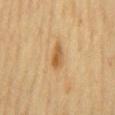notes = catalogued during a skin exam; not biopsied
imaging modality = 15 mm crop, total-body photography
location = the mid back
patient = female, approximately 55 years of age